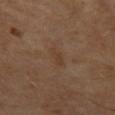workup — total-body-photography surveillance lesion; no biopsy
image source — total-body-photography crop, ~15 mm field of view
illumination — cross-polarized illumination
subject — male, aged 63 to 67
size — ≈3 mm